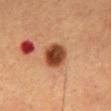<case>
<biopsy_status>not biopsied; imaged during a skin examination</biopsy_status>
<image>
  <source>total-body photography crop</source>
  <field_of_view_mm>15</field_of_view_mm>
</image>
<patient>
  <sex>male</sex>
  <age_approx>55</age_approx>
</patient>
<automated_metrics>
  <area_mm2_approx>9.0</area_mm2_approx>
  <eccentricity>0.55</eccentricity>
  <cielab_L>38</cielab_L>
  <cielab_a>23</cielab_a>
  <cielab_b>31</cielab_b>
  <vs_skin_darker_L>16.0</vs_skin_darker_L>
  <vs_skin_contrast_norm>12.5</vs_skin_contrast_norm>
</automated_metrics>
<site>abdomen</site>
</case>